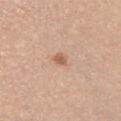follow-up: imaged on a skin check; not biopsied | lighting: white-light | image-analysis metrics: a footprint of about 5 mm², an eccentricity of roughly 0.7, and a shape-asymmetry score of about 0.3 (0 = symmetric); about 7 CIELAB-L* units darker than the surrounding skin and a lesion-to-skin contrast of about 4.5 (normalized; higher = more distinct); a detector confidence of about 100 out of 100 that the crop contains a lesion | subject: female, in their mid- to late 60s | anatomic site: the left upper arm | image source: ~15 mm crop, total-body skin-cancer survey.